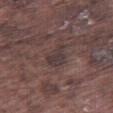Case summary:
- biopsy status · total-body-photography surveillance lesion; no biopsy
- lighting · white-light
- image · 15 mm crop, total-body photography
- lesion diameter · ≈3.5 mm
- patient · male, aged 73–77
- site · the right thigh